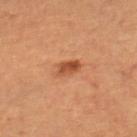follow-up: total-body-photography surveillance lesion; no biopsy | patient: female, aged 58 to 62 | diameter: ~3 mm (longest diameter) | imaging modality: ~15 mm tile from a whole-body skin photo | location: the right thigh | illumination: cross-polarized illumination.